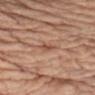This lesion was catalogued during total-body skin photography and was not selected for biopsy.
A female patient, roughly 65 years of age.
The lesion is on the left upper arm.
The tile uses white-light illumination.
Cropped from a total-body skin-imaging series; the visible field is about 15 mm.